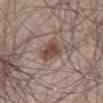| feature | finding |
|---|---|
| workup | catalogued during a skin exam; not biopsied |
| TBP lesion metrics | a lesion area of about 6 mm², an eccentricity of roughly 0.75, and a shape-asymmetry score of about 0.25 (0 = symmetric); a lesion color around L≈46 a*≈18 b*≈22 in CIELAB and a normalized border contrast of about 8.5; border irregularity of about 3 on a 0–10 scale, internal color variation of about 3 on a 0–10 scale, and peripheral color asymmetry of about 1; a nevus-likeness score of about 90/100 and lesion-presence confidence of about 100/100 |
| lesion diameter | about 3.5 mm |
| location | the right thigh |
| image source | 15 mm crop, total-body photography |
| illumination | white-light illumination |
| patient | male, about 55 years old |